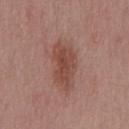Assessment: Recorded during total-body skin imaging; not selected for excision or biopsy. Image and clinical context: A region of skin cropped from a whole-body photographic capture, roughly 15 mm wide. The lesion's longest dimension is about 5.5 mm. Located on the chest. A male patient, approximately 40 years of age. An algorithmic analysis of the crop reported a lesion color around L≈46 a*≈23 b*≈25 in CIELAB, a lesion–skin lightness drop of about 9, and a lesion-to-skin contrast of about 7.5 (normalized; higher = more distinct). And it measured a nevus-likeness score of about 20/100. This is a white-light tile.